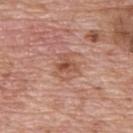biopsy status: total-body-photography surveillance lesion; no biopsy
illumination: white-light
image source: ~15 mm crop, total-body skin-cancer survey
anatomic site: the upper back
subject: male, in their 70s
diameter: about 3.5 mm
image-analysis metrics: a shape eccentricity near 0.6 and two-axis asymmetry of about 0.3; internal color variation of about 5 on a 0–10 scale; a nevus-likeness score of about 0/100 and a lesion-detection confidence of about 100/100